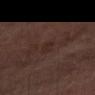Q: Was this lesion biopsied?
A: imaged on a skin check; not biopsied
Q: What is the imaging modality?
A: ~15 mm tile from a whole-body skin photo
Q: Illumination type?
A: white-light illumination
Q: How large is the lesion?
A: ~2.5 mm (longest diameter)
Q: What is the anatomic site?
A: the arm
Q: What are the patient's age and sex?
A: male, about 75 years old